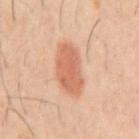workup — imaged on a skin check; not biopsied
size — ≈5.5 mm
illumination — cross-polarized illumination
site — the mid back
patient — male, aged 38–42
image — total-body-photography crop, ~15 mm field of view